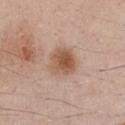biopsy status: no biopsy performed (imaged during a skin exam); lesion size: about 3.5 mm; site: the chest; patient: male, in their mid-30s; illumination: white-light; acquisition: 15 mm crop, total-body photography.